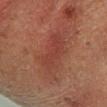notes: catalogued during a skin exam; not biopsied | image source: 15 mm crop, total-body photography | subject: female, aged around 50 | body site: the left lower leg.